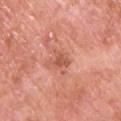Clinical impression:
Imaged during a routine full-body skin examination; the lesion was not biopsied and no histopathology is available.
Acquisition and patient details:
Located on the chest. The patient is a male aged 68–72. A close-up tile cropped from a whole-body skin photograph, about 15 mm across.